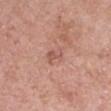- workup — no biopsy performed (imaged during a skin exam)
- lighting — white-light illumination
- diameter — ~2.5 mm (longest diameter)
- subject — female, approximately 40 years of age
- image source — total-body-photography crop, ~15 mm field of view
- site — the arm